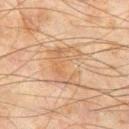Q: Was a biopsy performed?
A: total-body-photography surveillance lesion; no biopsy